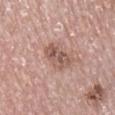Q: Is there a histopathology result?
A: total-body-photography surveillance lesion; no biopsy
Q: What is the anatomic site?
A: the right lower leg
Q: Patient demographics?
A: male, aged 73–77
Q: What is the imaging modality?
A: ~15 mm tile from a whole-body skin photo